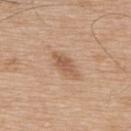Case summary:
• biopsy status · no biopsy performed (imaged during a skin exam)